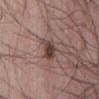Imaged during a routine full-body skin examination; the lesion was not biopsied and no histopathology is available.
Cropped from a total-body skin-imaging series; the visible field is about 15 mm.
The lesion is on the abdomen.
The patient is a male aged approximately 75.